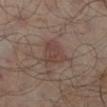Findings:
– follow-up — no biopsy performed (imaged during a skin exam)
– image-analysis metrics — a shape eccentricity near 0.6 and a symmetry-axis asymmetry near 0.35; an average lesion color of about L≈38 a*≈16 b*≈20 (CIELAB), about 6 CIELAB-L* units darker than the surrounding skin, and a lesion-to-skin contrast of about 6 (normalized; higher = more distinct); a border-irregularity index near 3.5/10 and a within-lesion color-variation index near 3/10
– lighting — cross-polarized illumination
– subject — male, about 65 years old
– location — the left lower leg
– lesion diameter — ~4 mm (longest diameter)
– image — total-body-photography crop, ~15 mm field of view